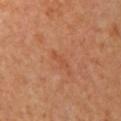Case summary:
* notes — imaged on a skin check; not biopsied
* subject — female, aged 63–67
* diameter — ~2.5 mm (longest diameter)
* acquisition — ~15 mm crop, total-body skin-cancer survey
* tile lighting — cross-polarized illumination
* anatomic site — the chest